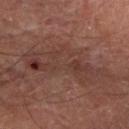Imaged during a routine full-body skin examination; the lesion was not biopsied and no histopathology is available. The lesion's longest dimension is about 9.5 mm. A close-up tile cropped from a whole-body skin photograph, about 15 mm across. A male subject aged around 70. The total-body-photography lesion software estimated a classifier nevus-likeness of about 0/100 and a detector confidence of about 70 out of 100 that the crop contains a lesion. The lesion is on the right forearm. Captured under cross-polarized illumination.